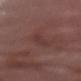Q: Was this lesion biopsied?
A: catalogued during a skin exam; not biopsied
Q: Illumination type?
A: white-light
Q: What is the imaging modality?
A: total-body-photography crop, ~15 mm field of view
Q: What did automated image analysis measure?
A: a lesion-detection confidence of about 90/100
Q: What are the patient's age and sex?
A: female, aged approximately 65
Q: What is the lesion's diameter?
A: about 3 mm
Q: Where on the body is the lesion?
A: the right forearm